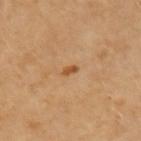workup=total-body-photography surveillance lesion; no biopsy | automated lesion analysis=a nevus-likeness score of about 90/100 | tile lighting=cross-polarized illumination | size=about 2 mm | subject=female, aged around 55 | anatomic site=the left upper arm | acquisition=total-body-photography crop, ~15 mm field of view.